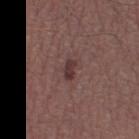The lesion was photographed on a routine skin check and not biopsied; there is no pathology result.
Automated image analysis of the tile measured a lesion area of about 3.5 mm² and a shape-asymmetry score of about 0.25 (0 = symmetric). The analysis additionally found a border-irregularity index near 2.5/10 and internal color variation of about 2.5 on a 0–10 scale.
The lesion is located on the left thigh.
This is a white-light tile.
A close-up tile cropped from a whole-body skin photograph, about 15 mm across.
Longest diameter approximately 2.5 mm.
The subject is a male about 45 years old.